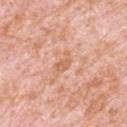Impression: Part of a total-body skin-imaging series; this lesion was reviewed on a skin check and was not flagged for biopsy. Context: A 15 mm close-up extracted from a 3D total-body photography capture. A male subject, approximately 80 years of age. The lesion is located on the upper back. This is a white-light tile.